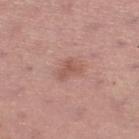notes = no biopsy performed (imaged during a skin exam)
acquisition = ~15 mm tile from a whole-body skin photo
site = the left lower leg
automated metrics = a lesion–skin lightness drop of about 8 and a normalized border contrast of about 6
lesion size = ~3 mm (longest diameter)
subject = female, aged around 55